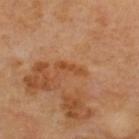biopsy status = total-body-photography surveillance lesion; no biopsy | lesion diameter = ~3.5 mm (longest diameter) | acquisition = 15 mm crop, total-body photography | location = the upper back | subject = male, aged 68–72.